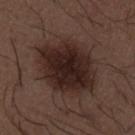{
  "automated_metrics": {
    "area_mm2_approx": 37.0,
    "eccentricity": 0.75,
    "shape_asymmetry": 0.15,
    "cielab_L": 25,
    "cielab_a": 15,
    "cielab_b": 18,
    "vs_skin_contrast_norm": 11.5,
    "border_irregularity_0_10": 2.5,
    "color_variation_0_10": 5.0,
    "peripheral_color_asymmetry": 1.5
  },
  "lesion_size": {
    "long_diameter_mm_approx": 9.0
  },
  "site": "mid back",
  "image": {
    "source": "total-body photography crop",
    "field_of_view_mm": 15
  },
  "patient": {
    "sex": "male",
    "age_approx": 50
  }
}